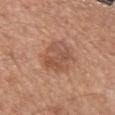The lesion was photographed on a routine skin check and not biopsied; there is no pathology result. On the arm. A female patient, about 50 years old. Measured at roughly 5 mm in maximum diameter. Captured under white-light illumination. A 15 mm close-up tile from a total-body photography series done for melanoma screening. Automated image analysis of the tile measured an average lesion color of about L≈53 a*≈22 b*≈30 (CIELAB) and a lesion–skin lightness drop of about 9. And it measured border irregularity of about 2.5 on a 0–10 scale, a color-variation rating of about 4.5/10, and radial color variation of about 1.5.